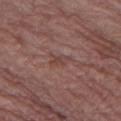* notes: no biopsy performed (imaged during a skin exam)
* automated metrics: internal color variation of about 0 on a 0–10 scale and a peripheral color-asymmetry measure near 0; a classifier nevus-likeness of about 0/100 and a detector confidence of about 80 out of 100 that the crop contains a lesion
* illumination: white-light illumination
* subject: female, aged approximately 65
* acquisition: total-body-photography crop, ~15 mm field of view
* site: the left thigh
* lesion size: about 2.5 mm